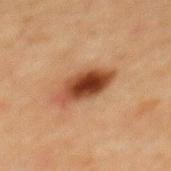{
  "biopsy_status": "not biopsied; imaged during a skin examination",
  "lighting": "cross-polarized",
  "patient": {
    "sex": "male",
    "age_approx": 50
  },
  "lesion_size": {
    "long_diameter_mm_approx": 5.5
  },
  "image": {
    "source": "total-body photography crop",
    "field_of_view_mm": 15
  },
  "site": "mid back"
}